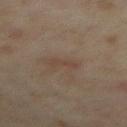Notes:
• follow-up — catalogued during a skin exam; not biopsied
• imaging modality — ~15 mm crop, total-body skin-cancer survey
• image-analysis metrics — a footprint of about 2.5 mm², a shape eccentricity near 0.95, and a symmetry-axis asymmetry near 0.3; a border-irregularity index near 4.5/10; a nevus-likeness score of about 0/100
• patient — female, about 35 years old
• lesion size — ~2.5 mm (longest diameter)
• illumination — cross-polarized illumination
• location — the right thigh